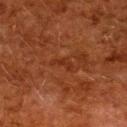• follow-up · catalogued during a skin exam; not biopsied
• image source · ~15 mm tile from a whole-body skin photo
• lesion diameter · about 3 mm
• automated metrics · a footprint of about 2.5 mm², an outline eccentricity of about 0.95 (0 = round, 1 = elongated), and two-axis asymmetry of about 0.6; a mean CIELAB color near L≈26 a*≈25 b*≈29, a lesion–skin lightness drop of about 5, and a lesion-to-skin contrast of about 5 (normalized; higher = more distinct); a border-irregularity index near 7/10 and a color-variation rating of about 0/10; a lesion-detection confidence of about 90/100
• body site · the back
• patient · female, aged around 50